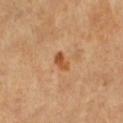No biopsy was performed on this lesion — it was imaged during a full skin examination and was not determined to be concerning.
The lesion is on the left lower leg.
A 15 mm close-up extracted from a 3D total-body photography capture.
A female patient in their mid-50s.